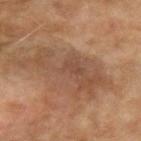| field | value |
|---|---|
| notes | total-body-photography surveillance lesion; no biopsy |
| tile lighting | cross-polarized illumination |
| patient | female, approximately 60 years of age |
| size | ≈9 mm |
| image-analysis metrics | a lesion area of about 22 mm², a shape eccentricity near 0.9, and two-axis asymmetry of about 0.5; a lesion color around L≈42 a*≈17 b*≈27 in CIELAB, a lesion–skin lightness drop of about 7, and a normalized border contrast of about 5.5; a border-irregularity rating of about 9/10 and a color-variation rating of about 3.5/10 |
| acquisition | 15 mm crop, total-body photography |
| anatomic site | the left forearm |